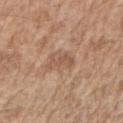This lesion was catalogued during total-body skin photography and was not selected for biopsy.
Measured at roughly 3 mm in maximum diameter.
A male patient, aged 68–72.
This is a white-light tile.
A lesion tile, about 15 mm wide, cut from a 3D total-body photograph.
The lesion-visualizer software estimated an area of roughly 4.5 mm², a shape eccentricity near 0.8, and two-axis asymmetry of about 0.35. The analysis additionally found a normalized lesion–skin contrast near 5.5. And it measured a classifier nevus-likeness of about 0/100 and a lesion-detection confidence of about 100/100.
Located on the right upper arm.